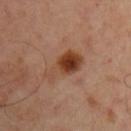The lesion was photographed on a routine skin check and not biopsied; there is no pathology result.
The patient is a male approximately 50 years of age.
This image is a 15 mm lesion crop taken from a total-body photograph.
From the chest.